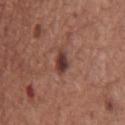• biopsy status — catalogued during a skin exam; not biopsied
• illumination — white-light illumination
• imaging modality — ~15 mm crop, total-body skin-cancer survey
• size — ~3 mm (longest diameter)
• patient — male, aged around 75
• location — the chest